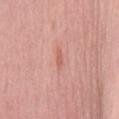{"biopsy_status": "not biopsied; imaged during a skin examination", "image": {"source": "total-body photography crop", "field_of_view_mm": 15}, "site": "mid back", "automated_metrics": {"area_mm2_approx": 2.0, "eccentricity": 0.95, "shape_asymmetry": 0.35, "color_variation_0_10": 0.0, "peripheral_color_asymmetry": 0.0, "nevus_likeness_0_100": 0, "lesion_detection_confidence_0_100": 100}, "patient": {"sex": "female", "age_approx": 60}}